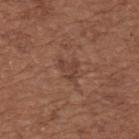Q: Was this lesion biopsied?
A: catalogued during a skin exam; not biopsied
Q: Patient demographics?
A: female, aged 63 to 67
Q: What is the imaging modality?
A: 15 mm crop, total-body photography
Q: Lesion location?
A: the upper back